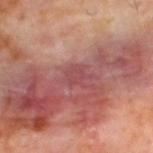The lesion was tiled from a total-body skin photograph and was not biopsied. The subject is a male aged 58 to 62. A 15 mm crop from a total-body photograph taken for skin-cancer surveillance. Imaged with cross-polarized lighting. Located on the upper back. About 3 mm across.